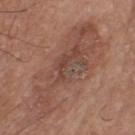Q: Was a biopsy performed?
A: total-body-photography surveillance lesion; no biopsy
Q: Automated lesion metrics?
A: a lesion color around L≈47 a*≈20 b*≈26 in CIELAB, about 7 CIELAB-L* units darker than the surrounding skin, and a lesion-to-skin contrast of about 6 (normalized; higher = more distinct); a nevus-likeness score of about 15/100
Q: What is the imaging modality?
A: total-body-photography crop, ~15 mm field of view
Q: What lighting was used for the tile?
A: white-light illumination
Q: Lesion location?
A: the chest
Q: Who is the patient?
A: male, approximately 75 years of age